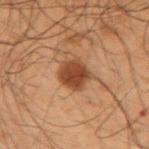{
  "biopsy_status": "not biopsied; imaged during a skin examination",
  "site": "right upper arm",
  "image": {
    "source": "total-body photography crop",
    "field_of_view_mm": 15
  },
  "patient": {
    "sex": "male",
    "age_approx": 50
  }
}